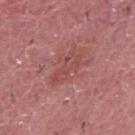notes: catalogued during a skin exam; not biopsied
patient: male, aged 38–42
location: the chest
image source: ~15 mm tile from a whole-body skin photo